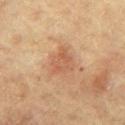Case summary:
* notes: imaged on a skin check; not biopsied
* diameter: ≈3 mm
* location: the front of the torso
* patient: male, aged approximately 65
* TBP lesion metrics: border irregularity of about 3 on a 0–10 scale, a within-lesion color-variation index near 3/10, and peripheral color asymmetry of about 1
* lighting: cross-polarized
* image: total-body-photography crop, ~15 mm field of view